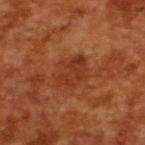No biopsy was performed on this lesion — it was imaged during a full skin examination and was not determined to be concerning.
Approximately 4 mm at its widest.
This is a cross-polarized tile.
A region of skin cropped from a whole-body photographic capture, roughly 15 mm wide.
A male subject about 65 years old.
The total-body-photography lesion software estimated about 7 CIELAB-L* units darker than the surrounding skin and a normalized lesion–skin contrast near 6.5. It also reported a classifier nevus-likeness of about 0/100 and a lesion-detection confidence of about 100/100.